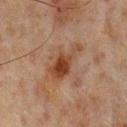notes — total-body-photography surveillance lesion; no biopsy | lesion size — about 5.5 mm | body site — the mid back | patient — male, aged approximately 65 | acquisition — 15 mm crop, total-body photography.